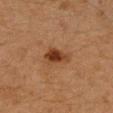notes: catalogued during a skin exam; not biopsied
acquisition: ~15 mm crop, total-body skin-cancer survey
body site: the arm
size: ~3.5 mm (longest diameter)
subject: female, aged 38 to 42
tile lighting: cross-polarized illumination
automated metrics: a symmetry-axis asymmetry near 0.3; a mean CIELAB color near L≈34 a*≈21 b*≈31, about 11 CIELAB-L* units darker than the surrounding skin, and a lesion-to-skin contrast of about 10 (normalized; higher = more distinct); border irregularity of about 2.5 on a 0–10 scale, a color-variation rating of about 4/10, and a peripheral color-asymmetry measure near 1.5; an automated nevus-likeness rating near 95 out of 100